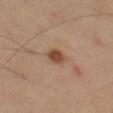biopsy status=no biopsy performed (imaged during a skin exam); location=the right leg; lesion size=≈2.5 mm; image=~15 mm crop, total-body skin-cancer survey; lighting=cross-polarized illumination; subject=female, aged 58–62.